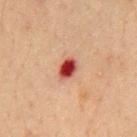Case summary:
* follow-up · no biopsy performed (imaged during a skin exam)
* image source · 15 mm crop, total-body photography
* subject · male, in their 50s
* size · ~3 mm (longest diameter)
* body site · the chest
* illumination · cross-polarized
* automated metrics · a border-irregularity rating of about 2/10, a within-lesion color-variation index near 4.5/10, and peripheral color asymmetry of about 1